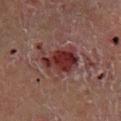The lesion was tiled from a total-body skin photograph and was not biopsied. A region of skin cropped from a whole-body photographic capture, roughly 15 mm wide. A male patient, approximately 55 years of age. On the left lower leg. Imaged with cross-polarized lighting. Measured at roughly 4.5 mm in maximum diameter.